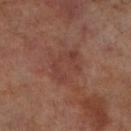Notes:
- follow-up: imaged on a skin check; not biopsied
- anatomic site: the right lower leg
- subject: male, approximately 70 years of age
- imaging modality: ~15 mm crop, total-body skin-cancer survey